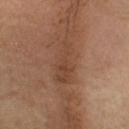The lesion was photographed on a routine skin check and not biopsied; there is no pathology result. Approximately 5.5 mm at its widest. This is a cross-polarized tile. A 15 mm crop from a total-body photograph taken for skin-cancer surveillance. An algorithmic analysis of the crop reported a border-irregularity index near 5.5/10 and peripheral color asymmetry of about 1. The analysis additionally found lesion-presence confidence of about 95/100. A female patient in their mid-60s. On the head or neck.